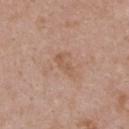| feature | finding |
|---|---|
| workup | no biopsy performed (imaged during a skin exam) |